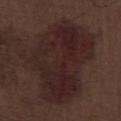Clinical impression: Captured during whole-body skin photography for melanoma surveillance; the lesion was not biopsied. Image and clinical context: Automated tile analysis of the lesion measured an area of roughly 60 mm² and a shape-asymmetry score of about 0.3 (0 = symmetric). It also reported about 7 CIELAB-L* units darker than the surrounding skin. The analysis additionally found border irregularity of about 4.5 on a 0–10 scale and a within-lesion color-variation index near 4.5/10. Approximately 10.5 mm at its widest. A region of skin cropped from a whole-body photographic capture, roughly 15 mm wide. On the leg. A male subject about 70 years old.